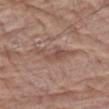Impression:
Captured during whole-body skin photography for melanoma surveillance; the lesion was not biopsied.
Image and clinical context:
A 15 mm crop from a total-body photograph taken for skin-cancer surveillance. A male patient, roughly 80 years of age. The lesion is on the left forearm.